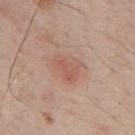This lesion was catalogued during total-body skin photography and was not selected for biopsy. Captured under white-light illumination. Cropped from a total-body skin-imaging series; the visible field is about 15 mm. About 4.5 mm across. The patient is a male aged around 70. From the upper back.